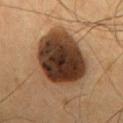patient: male, aged 53–57 | image-analysis metrics: a mean CIELAB color near L≈35 a*≈16 b*≈27, about 19 CIELAB-L* units darker than the surrounding skin, and a lesion-to-skin contrast of about 15.5 (normalized; higher = more distinct) | location: the chest | lesion diameter: ~8.5 mm (longest diameter) | imaging modality: 15 mm crop, total-body photography.